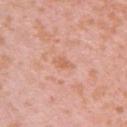The lesion was tiled from a total-body skin photograph and was not biopsied. A female subject, aged 38 to 42. A lesion tile, about 15 mm wide, cut from a 3D total-body photograph. On the chest.